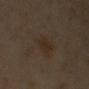Part of a total-body skin-imaging series; this lesion was reviewed on a skin check and was not flagged for biopsy.
Captured under cross-polarized illumination.
A 15 mm crop from a total-body photograph taken for skin-cancer surveillance.
The recorded lesion diameter is about 5.5 mm.
A male subject, aged approximately 65.
The lesion-visualizer software estimated a lesion area of about 10 mm², an outline eccentricity of about 0.9 (0 = round, 1 = elongated), and a shape-asymmetry score of about 0.35 (0 = symmetric). The software also gave a mean CIELAB color near L≈26 a*≈10 b*≈22, about 4 CIELAB-L* units darker than the surrounding skin, and a normalized lesion–skin contrast near 5.5. The software also gave an automated nevus-likeness rating near 5 out of 100 and a detector confidence of about 100 out of 100 that the crop contains a lesion.